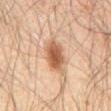Captured during whole-body skin photography for melanoma surveillance; the lesion was not biopsied.